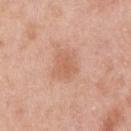A 15 mm crop from a total-body photograph taken for skin-cancer surveillance. The patient is a male approximately 45 years of age. The lesion is located on the upper back.